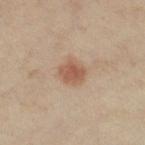{
  "biopsy_status": "not biopsied; imaged during a skin examination",
  "automated_metrics": {
    "area_mm2_approx": 6.5,
    "eccentricity": 0.45,
    "shape_asymmetry": 0.2,
    "cielab_L": 53,
    "cielab_a": 19,
    "cielab_b": 29,
    "vs_skin_darker_L": 10.0,
    "vs_skin_contrast_norm": 7.0,
    "color_variation_0_10": 2.5,
    "peripheral_color_asymmetry": 1.0
  },
  "site": "right thigh",
  "image": {
    "source": "total-body photography crop",
    "field_of_view_mm": 15
  },
  "lesion_size": {
    "long_diameter_mm_approx": 3.0
  },
  "patient": {
    "sex": "female",
    "age_approx": 35
  }
}